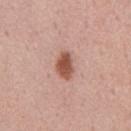Findings:
– biopsy status — no biopsy performed (imaged during a skin exam)
– illumination — white-light
– lesion diameter — about 3.5 mm
– patient — male, roughly 75 years of age
– imaging modality — ~15 mm crop, total-body skin-cancer survey
– automated lesion analysis — a lesion area of about 6 mm², an eccentricity of roughly 0.75, and two-axis asymmetry of about 0.25; a classifier nevus-likeness of about 100/100 and a lesion-detection confidence of about 100/100
– body site — the chest